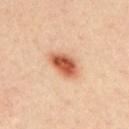Recorded during total-body skin imaging; not selected for excision or biopsy. Measured at roughly 4 mm in maximum diameter. Located on the upper back. A roughly 15 mm field-of-view crop from a total-body skin photograph. Automated image analysis of the tile measured an eccentricity of roughly 0.8 and a symmetry-axis asymmetry near 0.2. The analysis additionally found roughly 17 lightness units darker than nearby skin. The analysis additionally found a border-irregularity rating of about 2/10 and a color-variation rating of about 5.5/10. The analysis additionally found an automated nevus-likeness rating near 100 out of 100 and a detector confidence of about 100 out of 100 that the crop contains a lesion. This is a cross-polarized tile. A male subject aged 33–37.